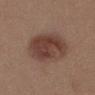{"biopsy_status": "not biopsied; imaged during a skin examination", "lesion_size": {"long_diameter_mm_approx": 5.5}, "site": "chest", "patient": {"sex": "female", "age_approx": 35}, "image": {"source": "total-body photography crop", "field_of_view_mm": 15}, "lighting": "white-light"}